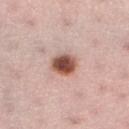biopsy status — total-body-photography surveillance lesion; no biopsy | illumination — white-light illumination | lesion diameter — ~3.5 mm (longest diameter) | site — the left lower leg | acquisition — ~15 mm crop, total-body skin-cancer survey | subject — female, about 35 years old.